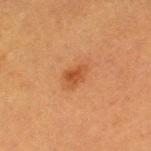<record>
  <biopsy_status>not biopsied; imaged during a skin examination</biopsy_status>
  <site>left thigh</site>
  <image>
    <source>total-body photography crop</source>
    <field_of_view_mm>15</field_of_view_mm>
  </image>
  <lighting>cross-polarized</lighting>
  <patient>
    <sex>female</sex>
    <age_approx>40</age_approx>
  </patient>
  <lesion_size>
    <long_diameter_mm_approx>3.0</long_diameter_mm_approx>
  </lesion_size>
</record>